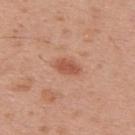Impression:
Imaged during a routine full-body skin examination; the lesion was not biopsied and no histopathology is available.
Image and clinical context:
A 15 mm close-up tile from a total-body photography series done for melanoma screening. The patient is a male approximately 30 years of age. An algorithmic analysis of the crop reported a lesion area of about 4.5 mm², an outline eccentricity of about 0.85 (0 = round, 1 = elongated), and a symmetry-axis asymmetry near 0.2. The software also gave a mean CIELAB color near L≈54 a*≈26 b*≈32 and a lesion-to-skin contrast of about 7 (normalized; higher = more distinct). The analysis additionally found a color-variation rating of about 1/10 and radial color variation of about 0.5. It also reported a classifier nevus-likeness of about 85/100 and a detector confidence of about 100 out of 100 that the crop contains a lesion. This is a white-light tile. The lesion is on the upper back. The lesion's longest dimension is about 3 mm.